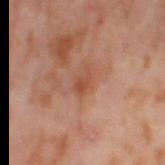Imaged during a routine full-body skin examination; the lesion was not biopsied and no histopathology is available.
A close-up tile cropped from a whole-body skin photograph, about 15 mm across.
The tile uses cross-polarized illumination.
The recorded lesion diameter is about 3 mm.
A female subject aged 53–57.
On the left thigh.